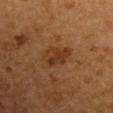Q: Was a biopsy performed?
A: total-body-photography surveillance lesion; no biopsy
Q: How was this image acquired?
A: ~15 mm tile from a whole-body skin photo
Q: Patient demographics?
A: male, about 55 years old
Q: Illumination type?
A: cross-polarized
Q: Lesion location?
A: the right upper arm
Q: What did automated image analysis measure?
A: about 7 CIELAB-L* units darker than the surrounding skin; a classifier nevus-likeness of about 35/100